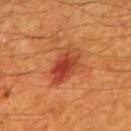This lesion was catalogued during total-body skin photography and was not selected for biopsy. The lesion is located on the mid back. A 15 mm close-up tile from a total-body photography series done for melanoma screening. This is a cross-polarized tile. A male subject, in their 60s. Approximately 5 mm at its widest.